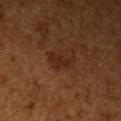– workup: total-body-photography surveillance lesion; no biopsy
– subject: female, aged 53–57
– illumination: cross-polarized
– size: ~3.5 mm (longest diameter)
– image source: ~15 mm tile from a whole-body skin photo
– location: the left upper arm